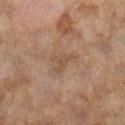{"biopsy_status": "not biopsied; imaged during a skin examination", "patient": {"sex": "female", "age_approx": 60}, "lighting": "cross-polarized", "image": {"source": "total-body photography crop", "field_of_view_mm": 15}, "site": "right lower leg", "automated_metrics": {"area_mm2_approx": 3.0, "eccentricity": 0.85, "shape_asymmetry": 0.4, "cielab_L": 43, "cielab_a": 15, "cielab_b": 27, "vs_skin_darker_L": 5.0, "border_irregularity_0_10": 4.5, "color_variation_0_10": 0.5, "peripheral_color_asymmetry": 0.0}}